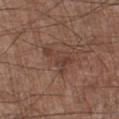subject = male, roughly 55 years of age
anatomic site = the left lower leg
image-analysis metrics = a footprint of about 7 mm² and a shape eccentricity near 0.8; a border-irregularity index near 7/10, a color-variation rating of about 2.5/10, and peripheral color asymmetry of about 1
lighting = white-light
lesion size = ~4 mm (longest diameter)
image = ~15 mm crop, total-body skin-cancer survey